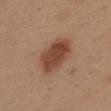Context:
The recorded lesion diameter is about 5.5 mm. Located on the upper back. The tile uses white-light illumination. The lesion-visualizer software estimated a border-irregularity rating of about 2/10, internal color variation of about 3 on a 0–10 scale, and peripheral color asymmetry of about 1. This image is a 15 mm lesion crop taken from a total-body photograph. A female subject, about 40 years old.